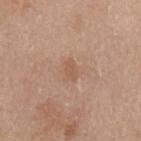Part of a total-body skin-imaging series; this lesion was reviewed on a skin check and was not flagged for biopsy. Longest diameter approximately 2.5 mm. Captured under white-light illumination. A 15 mm close-up tile from a total-body photography series done for melanoma screening. The subject is a male aged 78–82. The lesion is located on the left upper arm.